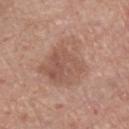<tbp_lesion>
<image>
  <source>total-body photography crop</source>
  <field_of_view_mm>15</field_of_view_mm>
</image>
<site>leg</site>
<lesion_size>
  <long_diameter_mm_approx>6.5</long_diameter_mm_approx>
</lesion_size>
<patient>
  <sex>male</sex>
  <age_approx>70</age_approx>
</patient>
</tbp_lesion>